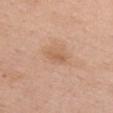Impression:
Part of a total-body skin-imaging series; this lesion was reviewed on a skin check and was not flagged for biopsy.
Acquisition and patient details:
On the chest. The lesion-visualizer software estimated an area of roughly 3.5 mm² and a symmetry-axis asymmetry near 0.25. And it measured a border-irregularity rating of about 2.5/10, internal color variation of about 2 on a 0–10 scale, and radial color variation of about 0.5. A female patient approximately 50 years of age. A roughly 15 mm field-of-view crop from a total-body skin photograph. About 2.5 mm across. This is a white-light tile.